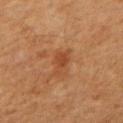Notes:
– automated metrics: a mean CIELAB color near L≈39 a*≈23 b*≈33, a lesion–skin lightness drop of about 7, and a normalized border contrast of about 6; border irregularity of about 4.5 on a 0–10 scale, internal color variation of about 1 on a 0–10 scale, and radial color variation of about 0
– image source: ~15 mm tile from a whole-body skin photo
– patient: female, about 40 years old
– lighting: cross-polarized
– body site: the arm